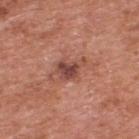{"biopsy_status": "not biopsied; imaged during a skin examination", "automated_metrics": {"vs_skin_contrast_norm": 8.0, "color_variation_0_10": 4.5, "peripheral_color_asymmetry": 1.5, "nevus_likeness_0_100": 10, "lesion_detection_confidence_0_100": 100}, "site": "upper back", "patient": {"sex": "male", "age_approx": 70}, "image": {"source": "total-body photography crop", "field_of_view_mm": 15}, "lighting": "white-light"}